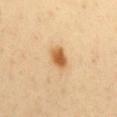Impression: The lesion was photographed on a routine skin check and not biopsied; there is no pathology result. Acquisition and patient details: Captured under cross-polarized illumination. The subject is a male aged approximately 40. The lesion-visualizer software estimated a footprint of about 5.5 mm², an outline eccentricity of about 0.75 (0 = round, 1 = elongated), and a shape-asymmetry score of about 0.2 (0 = symmetric). It also reported border irregularity of about 1.5 on a 0–10 scale, a within-lesion color-variation index near 4/10, and peripheral color asymmetry of about 1.5. The analysis additionally found a detector confidence of about 100 out of 100 that the crop contains a lesion. Cropped from a total-body skin-imaging series; the visible field is about 15 mm. About 3 mm across. The lesion is on the mid back.